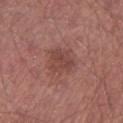notes = catalogued during a skin exam; not biopsied | location = the left lower leg | image = ~15 mm tile from a whole-body skin photo | subject = male, aged 53–57.